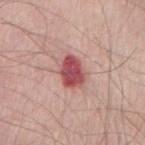{"biopsy_status": "not biopsied; imaged during a skin examination"}